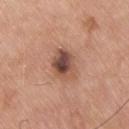The lesion was tiled from a total-body skin photograph and was not biopsied. The lesion's longest dimension is about 4 mm. The tile uses white-light illumination. An algorithmic analysis of the crop reported a lesion area of about 9.5 mm² and two-axis asymmetry of about 0.15. It also reported a mean CIELAB color near L≈50 a*≈21 b*≈27, roughly 14 lightness units darker than nearby skin, and a normalized border contrast of about 9.5. The software also gave a nevus-likeness score of about 30/100 and lesion-presence confidence of about 100/100. From the back. A male subject, aged 73 to 77. Cropped from a total-body skin-imaging series; the visible field is about 15 mm.